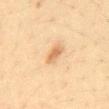Notes:
• notes — imaged on a skin check; not biopsied
• automated lesion analysis — a footprint of about 4 mm², a shape eccentricity near 0.85, and a shape-asymmetry score of about 0.25 (0 = symmetric); roughly 10 lightness units darker than nearby skin and a lesion-to-skin contrast of about 6.5 (normalized; higher = more distinct); a nevus-likeness score of about 75/100 and a detector confidence of about 100 out of 100 that the crop contains a lesion
• location — the abdomen
• patient — male, approximately 35 years of age
• illumination — cross-polarized illumination
• image source — total-body-photography crop, ~15 mm field of view